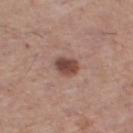Clinical impression: This lesion was catalogued during total-body skin photography and was not selected for biopsy. Background: A 15 mm crop from a total-body photograph taken for skin-cancer surveillance. The lesion is located on the right thigh. Captured under white-light illumination. A female subject aged around 50. The lesion's longest dimension is about 3 mm. Automated image analysis of the tile measured an area of roughly 6 mm² and a symmetry-axis asymmetry near 0.15. The analysis additionally found a border-irregularity index near 1.5/10, internal color variation of about 3.5 on a 0–10 scale, and radial color variation of about 1.